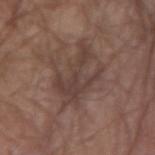Recorded during total-body skin imaging; not selected for excision or biopsy.
From the arm.
A close-up tile cropped from a whole-body skin photograph, about 15 mm across.
Approximately 6.5 mm at its widest.
Imaged with white-light lighting.
A male subject approximately 80 years of age.